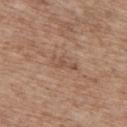biopsy_status: not biopsied; imaged during a skin examination
lesion_size:
  long_diameter_mm_approx: 3.0
image:
  source: total-body photography crop
  field_of_view_mm: 15
patient:
  sex: male
  age_approx: 70
lighting: white-light
site: back
automated_metrics:
  cielab_L: 51
  cielab_a: 19
  cielab_b: 28
  vs_skin_darker_L: 7.0
  vs_skin_contrast_norm: 5.5
  lesion_detection_confidence_0_100: 100